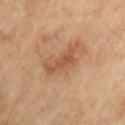automated lesion analysis: an area of roughly 6.5 mm² and a shape-asymmetry score of about 0.5 (0 = symmetric); a lesion color around L≈50 a*≈22 b*≈32 in CIELAB, roughly 8 lightness units darker than nearby skin, and a normalized border contrast of about 6; a nevus-likeness score of about 0/100 and a lesion-detection confidence of about 100/100
imaging modality: ~15 mm crop, total-body skin-cancer survey
subject: male, aged 63 to 67
lesion diameter: about 4.5 mm
lighting: cross-polarized illumination
location: the arm